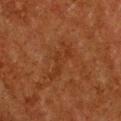Captured during whole-body skin photography for melanoma surveillance; the lesion was not biopsied.
The subject is a female aged 48 to 52.
A roughly 15 mm field-of-view crop from a total-body skin photograph.
The recorded lesion diameter is about 5 mm.
On the chest.